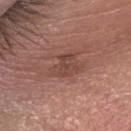follow-up — no biopsy performed (imaged during a skin exam)
location — the head or neck
image — total-body-photography crop, ~15 mm field of view
image-analysis metrics — a mean CIELAB color near L≈44 a*≈22 b*≈25, about 7 CIELAB-L* units darker than the surrounding skin, and a lesion-to-skin contrast of about 5.5 (normalized; higher = more distinct); border irregularity of about 4 on a 0–10 scale; an automated nevus-likeness rating near 0 out of 100 and a detector confidence of about 100 out of 100 that the crop contains a lesion
size — ≈3.5 mm
patient — male, aged around 70